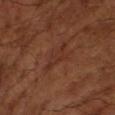Recorded during total-body skin imaging; not selected for excision or biopsy. A close-up tile cropped from a whole-body skin photograph, about 15 mm across. A male subject aged approximately 65. About 4.5 mm across.